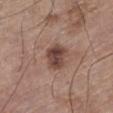{
  "biopsy_status": "not biopsied; imaged during a skin examination",
  "image": {
    "source": "total-body photography crop",
    "field_of_view_mm": 15
  },
  "site": "left lower leg",
  "patient": {
    "sex": "male",
    "age_approx": 65
  },
  "lighting": "white-light",
  "automated_metrics": {
    "area_mm2_approx": 7.5,
    "eccentricity": 0.6,
    "shape_asymmetry": 0.25,
    "border_irregularity_0_10": 2.5,
    "color_variation_0_10": 5.0,
    "peripheral_color_asymmetry": 2.0,
    "nevus_likeness_0_100": 70,
    "lesion_detection_confidence_0_100": 100
  }
}